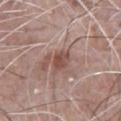{"biopsy_status": "not biopsied; imaged during a skin examination", "patient": {"sex": "male", "age_approx": 70}, "image": {"source": "total-body photography crop", "field_of_view_mm": 15}, "site": "front of the torso", "lighting": "white-light", "lesion_size": {"long_diameter_mm_approx": 2.5}, "automated_metrics": {"cielab_L": 49, "cielab_a": 20, "cielab_b": 25, "vs_skin_darker_L": 9.0, "vs_skin_contrast_norm": 7.5, "nevus_likeness_0_100": 5, "lesion_detection_confidence_0_100": 100}}